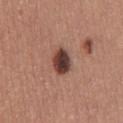The lesion was tiled from a total-body skin photograph and was not biopsied. About 3.5 mm across. The lesion is located on the mid back. The patient is a female in their 50s. An algorithmic analysis of the crop reported an average lesion color of about L≈40 a*≈21 b*≈23 (CIELAB) and a lesion–skin lightness drop of about 17. It also reported an automated nevus-likeness rating near 75 out of 100. A 15 mm crop from a total-body photograph taken for skin-cancer surveillance. Captured under white-light illumination.